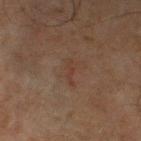workup: imaged on a skin check; not biopsied
diameter: about 3 mm
illumination: cross-polarized
subject: male, aged around 45
anatomic site: the left lower leg
image source: 15 mm crop, total-body photography